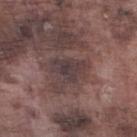The recorded lesion diameter is about 4 mm. A male patient in their mid- to late 70s. Cropped from a total-body skin-imaging series; the visible field is about 15 mm. The lesion is on the leg. This is a white-light tile.